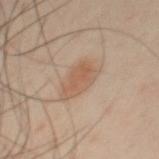Part of a total-body skin-imaging series; this lesion was reviewed on a skin check and was not flagged for biopsy. A male subject in their 50s. From the front of the torso. A 15 mm close-up extracted from a 3D total-body photography capture.